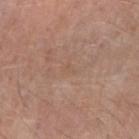Q: Is there a histopathology result?
A: catalogued during a skin exam; not biopsied